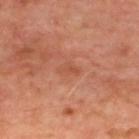The lesion was photographed on a routine skin check and not biopsied; there is no pathology result. On the upper back. Measured at roughly 3 mm in maximum diameter. A close-up tile cropped from a whole-body skin photograph, about 15 mm across. Automated tile analysis of the lesion measured a mean CIELAB color near L≈52 a*≈27 b*≈34, about 6 CIELAB-L* units darker than the surrounding skin, and a normalized lesion–skin contrast near 4.5. The analysis additionally found a border-irregularity rating of about 3.5/10, a color-variation rating of about 2/10, and radial color variation of about 0.5. A male patient aged 48 to 52.